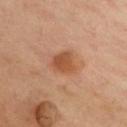Impression:
The lesion was photographed on a routine skin check and not biopsied; there is no pathology result.
Clinical summary:
A male subject, roughly 45 years of age. This is a cross-polarized tile. The lesion is on the chest. A 15 mm crop from a total-body photograph taken for skin-cancer surveillance. Automated tile analysis of the lesion measured a lesion-detection confidence of about 100/100.